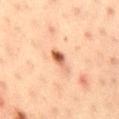Captured during whole-body skin photography for melanoma surveillance; the lesion was not biopsied. The lesion is on the right thigh. A male subject, approximately 35 years of age. Captured under cross-polarized illumination. Measured at roughly 3.5 mm in maximum diameter. The total-body-photography lesion software estimated an eccentricity of roughly 0.9 and a symmetry-axis asymmetry near 0.3. The software also gave a border-irregularity rating of about 3.5/10, a color-variation rating of about 6/10, and a peripheral color-asymmetry measure near 1.5. A 15 mm close-up extracted from a 3D total-body photography capture.